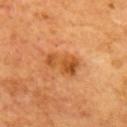biopsy_status: not biopsied; imaged during a skin examination
site: back
patient:
  sex: male
  age_approx: 65
image:
  source: total-body photography crop
  field_of_view_mm: 15